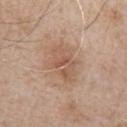Assessment:
Recorded during total-body skin imaging; not selected for excision or biopsy.
Background:
The lesion's longest dimension is about 5 mm. A male patient, aged 63 to 67. This is a white-light tile. A 15 mm close-up extracted from a 3D total-body photography capture. From the chest.